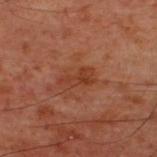{
  "biopsy_status": "not biopsied; imaged during a skin examination",
  "lighting": "cross-polarized",
  "image": {
    "source": "total-body photography crop",
    "field_of_view_mm": 15
  },
  "site": "upper back",
  "patient": {
    "sex": "male",
    "age_approx": 60
  },
  "lesion_size": {
    "long_diameter_mm_approx": 3.5
  }
}